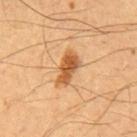Assessment: No biopsy was performed on this lesion — it was imaged during a full skin examination and was not determined to be concerning. Background: The lesion is on the chest. The subject is a male in their mid- to late 50s. A 15 mm close-up extracted from a 3D total-body photography capture.